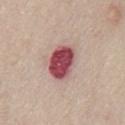lighting: white-light illumination
patient: male, aged around 65
image-analysis metrics: a color-variation rating of about 4.5/10 and a peripheral color-asymmetry measure near 1.5
acquisition: ~15 mm crop, total-body skin-cancer survey
site: the front of the torso